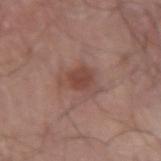workup: catalogued during a skin exam; not biopsied
image: total-body-photography crop, ~15 mm field of view
patient: male, about 65 years old
lighting: white-light
location: the right upper arm